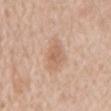The lesion was tiled from a total-body skin photograph and was not biopsied. The patient is a male roughly 80 years of age. The lesion is on the mid back. The tile uses white-light illumination. Cropped from a total-body skin-imaging series; the visible field is about 15 mm. The recorded lesion diameter is about 4 mm.